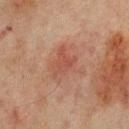notes: imaged on a skin check; not biopsied | lesion size: ≈4.5 mm | patient: male, roughly 65 years of age | image-analysis metrics: border irregularity of about 6 on a 0–10 scale and peripheral color asymmetry of about 1.5 | location: the back | image source: total-body-photography crop, ~15 mm field of view.